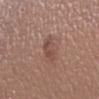| key | value |
|---|---|
| biopsy status | total-body-photography surveillance lesion; no biopsy |
| patient | female, aged 33–37 |
| diameter | about 3.5 mm |
| TBP lesion metrics | a mean CIELAB color near L≈48 a*≈20 b*≈24, about 8 CIELAB-L* units darker than the surrounding skin, and a normalized border contrast of about 6 |
| anatomic site | the right lower leg |
| imaging modality | 15 mm crop, total-body photography |